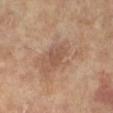The lesion was photographed on a routine skin check and not biopsied; there is no pathology result. The lesion is on the left lower leg. A female subject, aged around 75. A region of skin cropped from a whole-body photographic capture, roughly 15 mm wide. Approximately 3 mm at its widest. Captured under cross-polarized illumination.